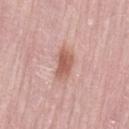biopsy status = catalogued during a skin exam; not biopsied | subject = female, aged 68 to 72 | image = 15 mm crop, total-body photography | location = the leg | illumination = white-light.